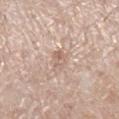Part of a total-body skin-imaging series; this lesion was reviewed on a skin check and was not flagged for biopsy. A 15 mm close-up tile from a total-body photography series done for melanoma screening. On the right lower leg. A female subject, aged approximately 55.